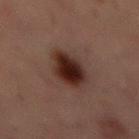Impression:
The lesion was photographed on a routine skin check and not biopsied; there is no pathology result.
Image and clinical context:
From the abdomen. A 15 mm close-up extracted from a 3D total-body photography capture. A male subject, approximately 55 years of age. Imaged with cross-polarized lighting. About 4.5 mm across.